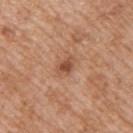Findings:
• notes — imaged on a skin check; not biopsied
• subject — male, aged around 60
• diameter — ~2.5 mm (longest diameter)
• image-analysis metrics — an area of roughly 3 mm², a shape eccentricity near 0.7, and two-axis asymmetry of about 0.3; internal color variation of about 2.5 on a 0–10 scale and radial color variation of about 1; a classifier nevus-likeness of about 60/100 and a lesion-detection confidence of about 100/100
• lighting — white-light
• anatomic site — the upper back
• acquisition — ~15 mm tile from a whole-body skin photo